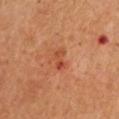* follow-up — catalogued during a skin exam; not biopsied
* illumination — cross-polarized
* imaging modality — total-body-photography crop, ~15 mm field of view
* patient — male, aged approximately 65
* location — the arm
* diameter — ≈3 mm